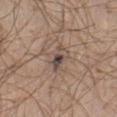Longest diameter approximately 3.5 mm.
The total-body-photography lesion software estimated an average lesion color of about L≈47 a*≈13 b*≈21 (CIELAB) and a normalized border contrast of about 8.5. It also reported an automated nevus-likeness rating near 5 out of 100 and lesion-presence confidence of about 95/100.
A male subject about 40 years old.
The tile uses white-light illumination.
A 15 mm crop from a total-body photograph taken for skin-cancer surveillance.
From the abdomen.